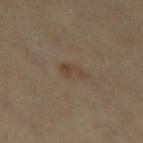Impression:
The lesion was photographed on a routine skin check and not biopsied; there is no pathology result.
Acquisition and patient details:
Longest diameter approximately 2.5 mm. A close-up tile cropped from a whole-body skin photograph, about 15 mm across. On the leg. The lesion-visualizer software estimated a footprint of about 3 mm², a shape eccentricity near 0.85, and a shape-asymmetry score of about 0.45 (0 = symmetric). And it measured a lesion color around L≈34 a*≈11 b*≈23 in CIELAB, about 5 CIELAB-L* units darker than the surrounding skin, and a normalized border contrast of about 6. The software also gave a within-lesion color-variation index near 1/10. A female subject in their 50s.